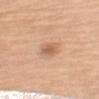Recorded during total-body skin imaging; not selected for excision or biopsy.
The lesion's longest dimension is about 3 mm.
Imaged with white-light lighting.
This image is a 15 mm lesion crop taken from a total-body photograph.
The subject is a female in their mid- to late 50s.
Located on the left upper arm.
An algorithmic analysis of the crop reported a footprint of about 4 mm², an outline eccentricity of about 0.85 (0 = round, 1 = elongated), and a symmetry-axis asymmetry near 0.25. The analysis additionally found a nevus-likeness score of about 40/100.